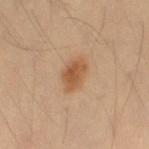Findings:
- biopsy status — no biopsy performed (imaged during a skin exam)
- subject — female, aged approximately 35
- site — the left thigh
- imaging modality — 15 mm crop, total-body photography